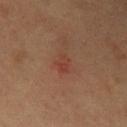This lesion was catalogued during total-body skin photography and was not selected for biopsy. A lesion tile, about 15 mm wide, cut from a 3D total-body photograph. The lesion-visualizer software estimated a border-irregularity index near 2.5/10, internal color variation of about 2.5 on a 0–10 scale, and a peripheral color-asymmetry measure near 1. The tile uses cross-polarized illumination. A male subject, aged 53 to 57. The lesion is on the chest.